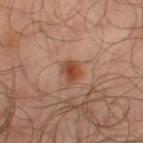Case summary:
• biopsy status — no biopsy performed (imaged during a skin exam)
• illumination — cross-polarized illumination
• anatomic site — the left thigh
• automated metrics — about 10 CIELAB-L* units darker than the surrounding skin and a normalized border contrast of about 9; an automated nevus-likeness rating near 95 out of 100 and a lesion-detection confidence of about 100/100
• subject — male, aged 63 to 67
• image source — 15 mm crop, total-body photography
• size — about 2.5 mm